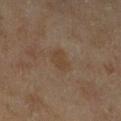{"biopsy_status": "not biopsied; imaged during a skin examination", "image": {"source": "total-body photography crop", "field_of_view_mm": 15}, "site": "left lower leg", "patient": {"sex": "female", "age_approx": 60}, "lesion_size": {"long_diameter_mm_approx": 3.0}, "automated_metrics": {"area_mm2_approx": 5.5, "shape_asymmetry": 0.2, "cielab_L": 37, "cielab_a": 13, "cielab_b": 25, "vs_skin_darker_L": 5.0, "vs_skin_contrast_norm": 5.5, "border_irregularity_0_10": 2.0, "color_variation_0_10": 1.5, "peripheral_color_asymmetry": 0.5, "nevus_likeness_0_100": 0, "lesion_detection_confidence_0_100": 100}}